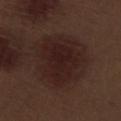The lesion was photographed on a routine skin check and not biopsied; there is no pathology result. Cropped from a total-body skin-imaging series; the visible field is about 15 mm. The lesion is on the right lower leg. The patient is a male in their 70s.